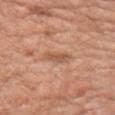Q: Is there a histopathology result?
A: imaged on a skin check; not biopsied
Q: Where on the body is the lesion?
A: the arm
Q: How was the tile lit?
A: white-light illumination
Q: What did automated image analysis measure?
A: a lesion color around L≈55 a*≈23 b*≈33 in CIELAB and a normalized border contrast of about 6.5; an automated nevus-likeness rating near 5 out of 100 and a detector confidence of about 100 out of 100 that the crop contains a lesion
Q: What kind of image is this?
A: ~15 mm crop, total-body skin-cancer survey
Q: Who is the patient?
A: male, aged 58 to 62